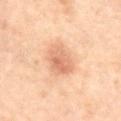<lesion>
  <biopsy_status>not biopsied; imaged during a skin examination</biopsy_status>
  <patient>
    <sex>male</sex>
    <age_approx>70</age_approx>
  </patient>
  <lighting>cross-polarized</lighting>
  <automated_metrics>
    <cielab_L>52</cielab_L>
    <cielab_a>18</cielab_a>
    <cielab_b>28</cielab_b>
    <vs_skin_darker_L>8.0</vs_skin_darker_L>
    <vs_skin_contrast_norm>6.0</vs_skin_contrast_norm>
  </automated_metrics>
  <site>abdomen</site>
  <image>
    <source>total-body photography crop</source>
    <field_of_view_mm>15</field_of_view_mm>
  </image>
  <lesion_size>
    <long_diameter_mm_approx>4.0</long_diameter_mm_approx>
  </lesion_size>
</lesion>